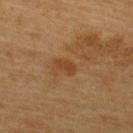biopsy status = no biopsy performed (imaged during a skin exam); subject = male, in their mid-60s; body site = the upper back; image source = ~15 mm tile from a whole-body skin photo; lesion size = ≈2.5 mm.